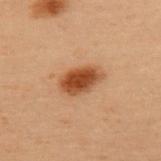Q: Was a biopsy performed?
A: total-body-photography surveillance lesion; no biopsy
Q: Lesion size?
A: ≈4.5 mm
Q: Automated lesion metrics?
A: a color-variation rating of about 4.5/10; a nevus-likeness score of about 100/100 and a lesion-detection confidence of about 100/100
Q: What kind of image is this?
A: ~15 mm crop, total-body skin-cancer survey
Q: Where on the body is the lesion?
A: the upper back
Q: How was the tile lit?
A: cross-polarized
Q: Who is the patient?
A: female, approximately 50 years of age